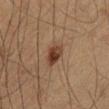Clinical impression:
Imaged during a routine full-body skin examination; the lesion was not biopsied and no histopathology is available.
Acquisition and patient details:
An algorithmic analysis of the crop reported roughly 10 lightness units darker than nearby skin and a normalized border contrast of about 9.5. Captured under cross-polarized illumination. A male subject, approximately 65 years of age. The recorded lesion diameter is about 3 mm. A region of skin cropped from a whole-body photographic capture, roughly 15 mm wide. Located on the abdomen.